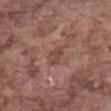Captured during whole-body skin photography for melanoma surveillance; the lesion was not biopsied.
A male patient, aged 73 to 77.
Automated tile analysis of the lesion measured a footprint of about 3.5 mm², an eccentricity of roughly 0.8, and two-axis asymmetry of about 0.25.
Located on the abdomen.
The lesion's longest dimension is about 2.5 mm.
A lesion tile, about 15 mm wide, cut from a 3D total-body photograph.